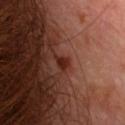Q: Was this lesion biopsied?
A: catalogued during a skin exam; not biopsied